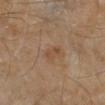Part of a total-body skin-imaging series; this lesion was reviewed on a skin check and was not flagged for biopsy.
A male patient aged approximately 55.
A lesion tile, about 15 mm wide, cut from a 3D total-body photograph.
Imaged with cross-polarized lighting.
The lesion is located on the right lower leg.
The lesion's longest dimension is about 2.5 mm.
The lesion-visualizer software estimated a shape eccentricity near 0.9 and a symmetry-axis asymmetry near 0.3. The analysis additionally found a border-irregularity index near 3.5/10, a within-lesion color-variation index near 0/10, and peripheral color asymmetry of about 0. It also reported an automated nevus-likeness rating near 0 out of 100 and lesion-presence confidence of about 100/100.